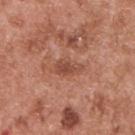biopsy status: total-body-photography surveillance lesion; no biopsy
lesion size: ≈3 mm
site: the upper back
acquisition: ~15 mm crop, total-body skin-cancer survey
lighting: white-light illumination
patient: male, about 55 years old